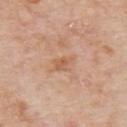Acquisition and patient details: A male patient, aged around 75. A region of skin cropped from a whole-body photographic capture, roughly 15 mm wide. About 3 mm across. The tile uses white-light illumination. Located on the upper back.